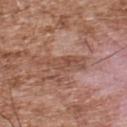Recorded during total-body skin imaging; not selected for excision or biopsy. Captured under white-light illumination. The lesion is on the back. A region of skin cropped from a whole-body photographic capture, roughly 15 mm wide. A male patient in their mid- to late 50s. The lesion-visualizer software estimated an average lesion color of about L≈50 a*≈21 b*≈28 (CIELAB), a lesion–skin lightness drop of about 8, and a normalized border contrast of about 6. And it measured a color-variation rating of about 3/10 and radial color variation of about 1. It also reported a classifier nevus-likeness of about 0/100 and a lesion-detection confidence of about 60/100. Approximately 5.5 mm at its widest.